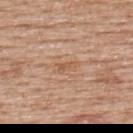Q: Was a biopsy performed?
A: catalogued during a skin exam; not biopsied
Q: Lesion location?
A: the upper back
Q: What is the imaging modality?
A: 15 mm crop, total-body photography
Q: Who is the patient?
A: male, roughly 60 years of age